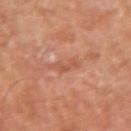Case summary:
• subject — male, aged 68 to 72
• body site — the right upper arm
• image source — 15 mm crop, total-body photography